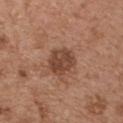| field | value |
|---|---|
| anatomic site | the chest |
| imaging modality | ~15 mm crop, total-body skin-cancer survey |
| subject | male, aged approximately 55 |
| diameter | about 3.5 mm |